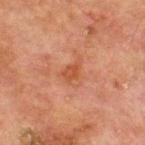No biopsy was performed on this lesion — it was imaged during a full skin examination and was not determined to be concerning. Imaged with cross-polarized lighting. Cropped from a whole-body photographic skin survey; the tile spans about 15 mm. From the chest. Automated tile analysis of the lesion measured a lesion color around L≈41 a*≈24 b*≈31 in CIELAB and a normalized lesion–skin contrast near 6.5. And it measured a classifier nevus-likeness of about 0/100 and lesion-presence confidence of about 100/100. The recorded lesion diameter is about 3 mm. A male patient, aged 63–67.